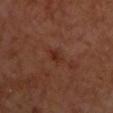  biopsy_status: not biopsied; imaged during a skin examination
  patient:
    sex: male
    age_approx: 70
  image:
    source: total-body photography crop
    field_of_view_mm: 15
  site: chest
  automated_metrics:
    eccentricity: 0.7
    shape_asymmetry: 0.25
    color_variation_0_10: 3.5
    nevus_likeness_0_100: 0